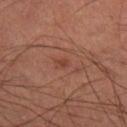location = the right thigh
size = about 1 mm
tile lighting = cross-polarized illumination
image = ~15 mm crop, total-body skin-cancer survey
patient = male, roughly 60 years of age